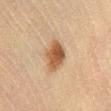biopsy status: total-body-photography surveillance lesion; no biopsy.